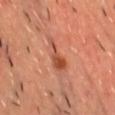Assessment: The lesion was tiled from a total-body skin photograph and was not biopsied. Context: Located on the chest. A region of skin cropped from a whole-body photographic capture, roughly 15 mm wide. Imaged with cross-polarized lighting. Automated image analysis of the tile measured a shape eccentricity near 0.95 and a shape-asymmetry score of about 0.5 (0 = symmetric). It also reported roughly 10 lightness units darker than nearby skin and a lesion-to-skin contrast of about 7 (normalized; higher = more distinct). The analysis additionally found a classifier nevus-likeness of about 60/100 and a detector confidence of about 100 out of 100 that the crop contains a lesion. The patient is a male in their mid-40s.